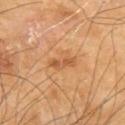Notes:
* notes — total-body-photography surveillance lesion; no biopsy
* imaging modality — ~15 mm tile from a whole-body skin photo
* patient — male, roughly 65 years of age
* site — the left upper arm
* lesion diameter — ~3 mm (longest diameter)
* lighting — cross-polarized